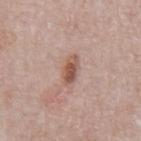The lesion was photographed on a routine skin check and not biopsied; there is no pathology result. This is a white-light tile. About 3.5 mm across. A male patient in their 70s. The lesion is located on the chest. A 15 mm close-up extracted from a 3D total-body photography capture.